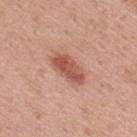workup: total-body-photography surveillance lesion; no biopsy | tile lighting: white-light illumination | image source: ~15 mm tile from a whole-body skin photo | patient: male, aged around 40 | site: the upper back.